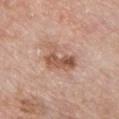The lesion was tiled from a total-body skin photograph and was not biopsied. A 15 mm close-up tile from a total-body photography series done for melanoma screening. A male subject aged 58 to 62. The total-body-photography lesion software estimated an average lesion color of about L≈56 a*≈21 b*≈29 (CIELAB), about 11 CIELAB-L* units darker than the surrounding skin, and a lesion-to-skin contrast of about 7.5 (normalized; higher = more distinct). It also reported border irregularity of about 4.5 on a 0–10 scale, internal color variation of about 7.5 on a 0–10 scale, and a peripheral color-asymmetry measure near 3. It also reported a lesion-detection confidence of about 100/100. From the front of the torso. Captured under white-light illumination.